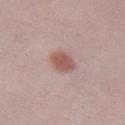Q: Is there a histopathology result?
A: imaged on a skin check; not biopsied
Q: How was the tile lit?
A: white-light
Q: Who is the patient?
A: male, roughly 30 years of age
Q: How was this image acquired?
A: 15 mm crop, total-body photography
Q: What is the lesion's diameter?
A: ≈3 mm